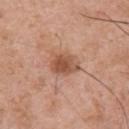follow-up: catalogued during a skin exam; not biopsied | image-analysis metrics: a footprint of about 7.5 mm², an outline eccentricity of about 0.7 (0 = round, 1 = elongated), and two-axis asymmetry of about 0.2 | subject: male, aged approximately 55 | body site: the left upper arm | illumination: white-light | acquisition: total-body-photography crop, ~15 mm field of view.